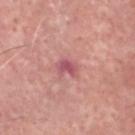Impression:
Captured during whole-body skin photography for melanoma surveillance; the lesion was not biopsied.